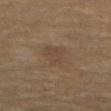| feature | finding |
|---|---|
| image | total-body-photography crop, ~15 mm field of view |
| lesion diameter | ≈4 mm |
| anatomic site | the abdomen |
| subject | male, aged 63–67 |
| illumination | cross-polarized illumination |